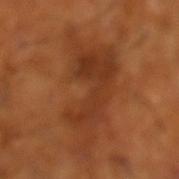notes = total-body-photography surveillance lesion; no biopsy | subject = male, aged approximately 65 | location = the left lower leg | acquisition = 15 mm crop, total-body photography | lesion diameter = ~10.5 mm (longest diameter).